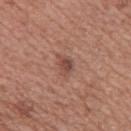Q: Was a biopsy performed?
A: imaged on a skin check; not biopsied
Q: Illumination type?
A: white-light
Q: What did automated image analysis measure?
A: a lesion area of about 3.5 mm², a shape eccentricity near 0.65, and a symmetry-axis asymmetry near 0.35; a nevus-likeness score of about 25/100 and a detector confidence of about 100 out of 100 that the crop contains a lesion
Q: What is the imaging modality?
A: 15 mm crop, total-body photography
Q: Who is the patient?
A: female, approximately 40 years of age
Q: Lesion size?
A: ≈2.5 mm
Q: Lesion location?
A: the upper back